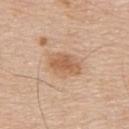No biopsy was performed on this lesion — it was imaged during a full skin examination and was not determined to be concerning. The recorded lesion diameter is about 4 mm. Automated tile analysis of the lesion measured a footprint of about 9.5 mm², an eccentricity of roughly 0.75, and two-axis asymmetry of about 0.15. And it measured a peripheral color-asymmetry measure near 1. And it measured a nevus-likeness score of about 50/100 and a detector confidence of about 100 out of 100 that the crop contains a lesion. The tile uses white-light illumination. A male patient, aged 78 to 82. A lesion tile, about 15 mm wide, cut from a 3D total-body photograph. From the upper back.